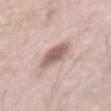automated_metrics:
  area_mm2_approx: 9.0
  shape_asymmetry: 0.2
  nevus_likeness_0_100: 80
image:
  source: total-body photography crop
  field_of_view_mm: 15
lesion_size:
  long_diameter_mm_approx: 4.0
lighting: white-light
site: chest
patient:
  sex: male
  age_approx: 70